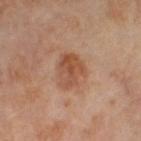Part of a total-body skin-imaging series; this lesion was reviewed on a skin check and was not flagged for biopsy. The lesion-visualizer software estimated a within-lesion color-variation index near 5.5/10 and radial color variation of about 2. And it measured an automated nevus-likeness rating near 0 out of 100. The patient is a female in their mid- to late 50s. About 4 mm across. This image is a 15 mm lesion crop taken from a total-body photograph. On the left thigh.